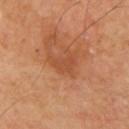Case summary:
- follow-up · imaged on a skin check; not biopsied
- site · the right upper arm
- image source · 15 mm crop, total-body photography